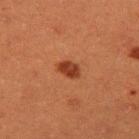The lesion was tiled from a total-body skin photograph and was not biopsied. A roughly 15 mm field-of-view crop from a total-body skin photograph. Automated tile analysis of the lesion measured a mean CIELAB color near L≈33 a*≈26 b*≈31, about 11 CIELAB-L* units darker than the surrounding skin, and a normalized lesion–skin contrast near 9.5. It also reported border irregularity of about 1.5 on a 0–10 scale, a color-variation rating of about 2/10, and radial color variation of about 0.5. A male subject, in their 40s. The recorded lesion diameter is about 3 mm. Imaged with cross-polarized lighting. From the left upper arm.